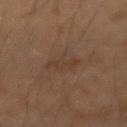A 15 mm close-up extracted from a 3D total-body photography capture.
The tile uses cross-polarized illumination.
The lesion's longest dimension is about 3.5 mm.
Automated tile analysis of the lesion measured a border-irregularity rating of about 5/10 and a peripheral color-asymmetry measure near 0.5.
On the right forearm.
A male patient aged 68 to 72.